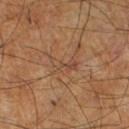Q: Is there a histopathology result?
A: no biopsy performed (imaged during a skin exam)
Q: What is the anatomic site?
A: the leg
Q: What did automated image analysis measure?
A: a footprint of about 3.5 mm²
Q: What are the patient's age and sex?
A: male, in their mid- to late 60s
Q: What kind of image is this?
A: total-body-photography crop, ~15 mm field of view